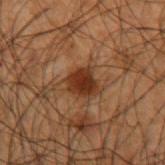Findings:
– biopsy status — catalogued during a skin exam; not biopsied
– lighting — cross-polarized
– diameter — ~3 mm (longest diameter)
– location — the left forearm
– subject — male, aged 48 to 52
– image — ~15 mm crop, total-body skin-cancer survey